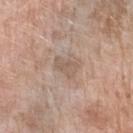Q: Was this lesion biopsied?
A: catalogued during a skin exam; not biopsied
Q: How was this image acquired?
A: total-body-photography crop, ~15 mm field of view
Q: Who is the patient?
A: female, aged around 75
Q: What lighting was used for the tile?
A: white-light
Q: What is the anatomic site?
A: the right forearm